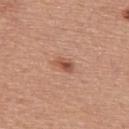Imaged during a routine full-body skin examination; the lesion was not biopsied and no histopathology is available. From the back. Captured under white-light illumination. A close-up tile cropped from a whole-body skin photograph, about 15 mm across. A male subject, aged around 55.